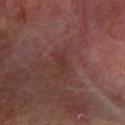follow-up: catalogued during a skin exam; not biopsied
imaging modality: total-body-photography crop, ~15 mm field of view
subject: male, approximately 65 years of age
body site: the left forearm